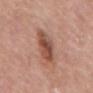A male patient, in their 80s. From the mid back. The lesion-visualizer software estimated an area of roughly 11 mm², a shape eccentricity near 0.9, and a shape-asymmetry score of about 0.2 (0 = symmetric). It also reported a mean CIELAB color near L≈50 a*≈23 b*≈28 and a lesion–skin lightness drop of about 12. The software also gave a border-irregularity index near 2.5/10 and a color-variation rating of about 6/10. The analysis additionally found a classifier nevus-likeness of about 80/100. A lesion tile, about 15 mm wide, cut from a 3D total-body photograph.